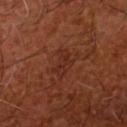This lesion was catalogued during total-body skin photography and was not selected for biopsy.
Captured under cross-polarized illumination.
A 15 mm crop from a total-body photograph taken for skin-cancer surveillance.
A male patient, aged 63 to 67.
An algorithmic analysis of the crop reported an area of roughly 6 mm² and a shape-asymmetry score of about 0.4 (0 = symmetric).
Longest diameter approximately 3.5 mm.